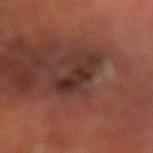Part of a total-body skin-imaging series; this lesion was reviewed on a skin check and was not flagged for biopsy. A close-up tile cropped from a whole-body skin photograph, about 15 mm across. Measured at roughly 5 mm in maximum diameter. A male patient, aged 63–67. From the left arm.